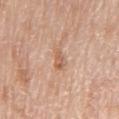Captured during whole-body skin photography for melanoma surveillance; the lesion was not biopsied.
Automated image analysis of the tile measured an area of roughly 3.5 mm². And it measured a border-irregularity rating of about 4/10 and peripheral color asymmetry of about 0.
Approximately 3 mm at its widest.
A 15 mm close-up tile from a total-body photography series done for melanoma screening.
Captured under white-light illumination.
On the chest.
A male patient aged around 80.